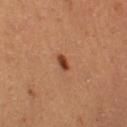Clinical impression:
The lesion was tiled from a total-body skin photograph and was not biopsied.